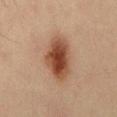Q: Was a biopsy performed?
A: total-body-photography surveillance lesion; no biopsy
Q: How was the tile lit?
A: cross-polarized
Q: What is the imaging modality?
A: ~15 mm tile from a whole-body skin photo
Q: Lesion size?
A: ~6 mm (longest diameter)
Q: Patient demographics?
A: male, approximately 35 years of age
Q: Where on the body is the lesion?
A: the mid back